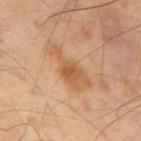{"biopsy_status": "not biopsied; imaged during a skin examination", "patient": {"sex": "male", "age_approx": 40}, "lighting": "cross-polarized", "site": "mid back", "lesion_size": {"long_diameter_mm_approx": 6.0}, "automated_metrics": {"eccentricity": 0.95, "shape_asymmetry": 0.55, "nevus_likeness_0_100": 0, "lesion_detection_confidence_0_100": 100}, "image": {"source": "total-body photography crop", "field_of_view_mm": 15}}